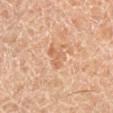| feature | finding |
|---|---|
| workup | imaged on a skin check; not biopsied |
| acquisition | total-body-photography crop, ~15 mm field of view |
| site | the right lower leg |
| illumination | cross-polarized |
| subject | male, about 55 years old |
| size | ~3 mm (longest diameter) |